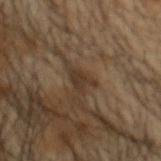Assessment:
Part of a total-body skin-imaging series; this lesion was reviewed on a skin check and was not flagged for biopsy.
Background:
The lesion is located on the head or neck. A roughly 15 mm field-of-view crop from a total-body skin photograph. The subject is a male aged 53–57.